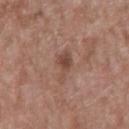Assessment:
The lesion was tiled from a total-body skin photograph and was not biopsied.
Clinical summary:
The total-body-photography lesion software estimated an area of roughly 6 mm². It also reported a mean CIELAB color near L≈47 a*≈19 b*≈25, roughly 8 lightness units darker than nearby skin, and a normalized lesion–skin contrast near 6.5. It also reported a border-irregularity index near 4/10, a within-lesion color-variation index near 2.5/10, and peripheral color asymmetry of about 0.5. The software also gave a nevus-likeness score of about 0/100. The patient is a male aged 48 to 52. Cropped from a whole-body photographic skin survey; the tile spans about 15 mm. Longest diameter approximately 4 mm. From the mid back. This is a white-light tile.